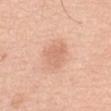The lesion was photographed on a routine skin check and not biopsied; there is no pathology result. A 15 mm close-up extracted from a 3D total-body photography capture. Automated image analysis of the tile measured a lesion color around L≈67 a*≈23 b*≈33 in CIELAB, a lesion–skin lightness drop of about 8, and a lesion-to-skin contrast of about 5 (normalized; higher = more distinct). On the front of the torso. Measured at roughly 3 mm in maximum diameter. The tile uses white-light illumination. A male patient, aged around 70.